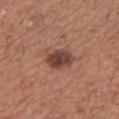follow-up = total-body-photography surveillance lesion; no biopsy | lighting = white-light | automated lesion analysis = a footprint of about 7.5 mm², a shape eccentricity near 0.8, and two-axis asymmetry of about 0.2; a border-irregularity index near 2/10, a within-lesion color-variation index near 3.5/10, and peripheral color asymmetry of about 1 | image source = 15 mm crop, total-body photography | site = the chest | diameter = ≈4 mm | patient = male, in their mid- to late 70s.